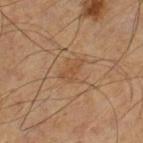workup: imaged on a skin check; not biopsied | subject: male, roughly 60 years of age | illumination: cross-polarized illumination | size: about 3 mm | imaging modality: ~15 mm crop, total-body skin-cancer survey | site: the left lower leg.